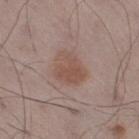| feature | finding |
|---|---|
| workup | no biopsy performed (imaged during a skin exam) |
| acquisition | 15 mm crop, total-body photography |
| location | the right thigh |
| lesion diameter | about 4 mm |
| subject | male, approximately 55 years of age |
| tile lighting | white-light illumination |